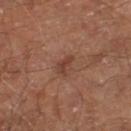follow-up = catalogued during a skin exam; not biopsied
body site = the right lower leg
acquisition = 15 mm crop, total-body photography
patient = male, in their mid-60s